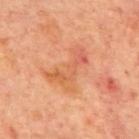No biopsy was performed on this lesion — it was imaged during a full skin examination and was not determined to be concerning.
The tile uses cross-polarized illumination.
The lesion is on the mid back.
A 15 mm crop from a total-body photograph taken for skin-cancer surveillance.
The subject is a male in their 70s.